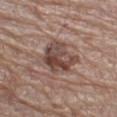Captured during whole-body skin photography for melanoma surveillance; the lesion was not biopsied.
From the left thigh.
Longest diameter approximately 5 mm.
This is a white-light tile.
A male subject aged 63–67.
A 15 mm close-up tile from a total-body photography series done for melanoma screening.